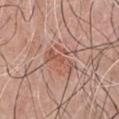biopsy status: no biopsy performed (imaged during a skin exam); subject: male, aged 68–72; image-analysis metrics: a shape eccentricity near 0.95 and a shape-asymmetry score of about 0.35 (0 = symmetric); image source: ~15 mm crop, total-body skin-cancer survey; illumination: white-light; site: the chest; size: about 4 mm.